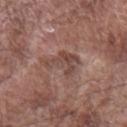Impression: The lesion was photographed on a routine skin check and not biopsied; there is no pathology result. Context: A male subject, aged 73 to 77. Captured under white-light illumination. From the left forearm. A 15 mm crop from a total-body photograph taken for skin-cancer surveillance. Longest diameter approximately 4 mm.